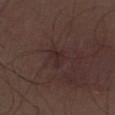Impression:
Part of a total-body skin-imaging series; this lesion was reviewed on a skin check and was not flagged for biopsy.
Acquisition and patient details:
Imaged with white-light lighting. The lesion is on the right thigh. A region of skin cropped from a whole-body photographic capture, roughly 15 mm wide. A male subject, about 30 years old.